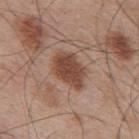| key | value |
|---|---|
| follow-up | no biopsy performed (imaged during a skin exam) |
| patient | male, roughly 55 years of age |
| automated lesion analysis | a footprint of about 11 mm², an eccentricity of roughly 0.75, and a shape-asymmetry score of about 0.15 (0 = symmetric); a mean CIELAB color near L≈46 a*≈21 b*≈27, a lesion–skin lightness drop of about 12, and a normalized border contrast of about 9.5; border irregularity of about 2 on a 0–10 scale, internal color variation of about 2.5 on a 0–10 scale, and a peripheral color-asymmetry measure near 1 |
| acquisition | ~15 mm tile from a whole-body skin photo |
| lighting | white-light illumination |
| lesion diameter | ~5 mm (longest diameter) |
| location | the mid back |